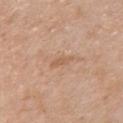Recorded during total-body skin imaging; not selected for excision or biopsy. The tile uses white-light illumination. The patient is a female aged around 55. Automated tile analysis of the lesion measured an area of roughly 2.5 mm², a shape eccentricity near 0.9, and a symmetry-axis asymmetry near 0.35. It also reported a mean CIELAB color near L≈59 a*≈20 b*≈33 and a lesion–skin lightness drop of about 7. The software also gave a border-irregularity rating of about 4/10 and a peripheral color-asymmetry measure near 0. The analysis additionally found a classifier nevus-likeness of about 0/100 and a detector confidence of about 100 out of 100 that the crop contains a lesion. A close-up tile cropped from a whole-body skin photograph, about 15 mm across. Approximately 3 mm at its widest. The lesion is on the chest.